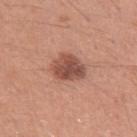Recorded during total-body skin imaging; not selected for excision or biopsy. The recorded lesion diameter is about 4 mm. A 15 mm close-up tile from a total-body photography series done for melanoma screening. The lesion is on the left upper arm. Imaged with white-light lighting. The subject is a male aged approximately 30.